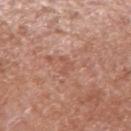Impression:
Captured during whole-body skin photography for melanoma surveillance; the lesion was not biopsied.
Image and clinical context:
Approximately 3 mm at its widest. The tile uses white-light illumination. On the arm. A 15 mm close-up extracted from a 3D total-body photography capture. The patient is a male in their mid- to late 60s. An algorithmic analysis of the crop reported a lesion area of about 2.5 mm², an eccentricity of roughly 0.85, and a symmetry-axis asymmetry near 0.65. It also reported a mean CIELAB color near L≈54 a*≈23 b*≈29, a lesion–skin lightness drop of about 6, and a normalized lesion–skin contrast near 4.5. The software also gave a border-irregularity index near 6.5/10, a within-lesion color-variation index near 0/10, and peripheral color asymmetry of about 0. It also reported a nevus-likeness score of about 0/100 and lesion-presence confidence of about 85/100.